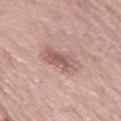Q: Is there a histopathology result?
A: total-body-photography surveillance lesion; no biopsy
Q: What is the anatomic site?
A: the left thigh
Q: What lighting was used for the tile?
A: white-light illumination
Q: What kind of image is this?
A: ~15 mm tile from a whole-body skin photo
Q: Lesion size?
A: about 4.5 mm
Q: Patient demographics?
A: female, aged 58 to 62